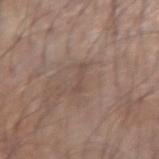Approximately 3 mm at its widest. Automated image analysis of the tile measured a lesion area of about 2.5 mm². It also reported a lesion color around L≈48 a*≈15 b*≈23 in CIELAB, a lesion–skin lightness drop of about 6, and a normalized lesion–skin contrast near 4.5. The analysis additionally found border irregularity of about 5.5 on a 0–10 scale, a color-variation rating of about 0/10, and a peripheral color-asymmetry measure near 0. The tile uses white-light illumination. A region of skin cropped from a whole-body photographic capture, roughly 15 mm wide. The lesion is located on the left forearm. The subject is a male aged approximately 70.